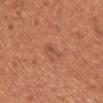Clinical impression: No biopsy was performed on this lesion — it was imaged during a full skin examination and was not determined to be concerning. Image and clinical context: The lesion is located on the front of the torso. The tile uses white-light illumination. A 15 mm close-up extracted from a 3D total-body photography capture. The lesion's longest dimension is about 3 mm. A female patient, aged approximately 50.